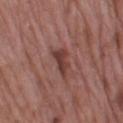| field | value |
|---|---|
| follow-up | no biopsy performed (imaged during a skin exam) |
| acquisition | ~15 mm crop, total-body skin-cancer survey |
| illumination | white-light illumination |
| automated metrics | an area of roughly 5.5 mm² and a shape-asymmetry score of about 0.35 (0 = symmetric); a lesion color around L≈40 a*≈23 b*≈23 in CIELAB and a lesion-to-skin contrast of about 8 (normalized; higher = more distinct); a nevus-likeness score of about 0/100 and a lesion-detection confidence of about 100/100 |
| lesion size | ~3.5 mm (longest diameter) |
| subject | female, roughly 70 years of age |
| body site | the leg |